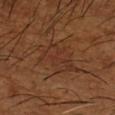Captured during whole-body skin photography for melanoma surveillance; the lesion was not biopsied. The recorded lesion diameter is about 5 mm. Located on the left forearm. A male subject, approximately 65 years of age. The tile uses cross-polarized illumination. Cropped from a total-body skin-imaging series; the visible field is about 15 mm.